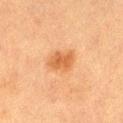{"patient": {"sex": "female", "age_approx": 55}, "automated_metrics": {"vs_skin_darker_L": 9.0, "nevus_likeness_0_100": 90}, "site": "right thigh", "lesion_size": {"long_diameter_mm_approx": 3.5}, "image": {"source": "total-body photography crop", "field_of_view_mm": 15}, "lighting": "cross-polarized"}